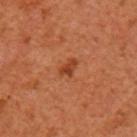workup: imaged on a skin check; not biopsied
imaging modality: ~15 mm tile from a whole-body skin photo
lesion diameter: ~2.5 mm (longest diameter)
site: the left upper arm
subject: male, in their mid-40s
illumination: cross-polarized illumination
TBP lesion metrics: an eccentricity of roughly 0.75; a nevus-likeness score of about 75/100 and lesion-presence confidence of about 100/100